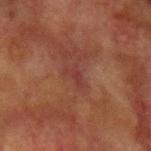Notes:
– notes: no biopsy performed (imaged during a skin exam)
– body site: the left upper arm
– imaging modality: total-body-photography crop, ~15 mm field of view
– subject: male, about 75 years old
– size: about 2.5 mm
– image-analysis metrics: a lesion color around L≈31 a*≈23 b*≈22 in CIELAB and about 5 CIELAB-L* units darker than the surrounding skin; a border-irregularity index near 4.5/10, a within-lesion color-variation index near 1/10, and peripheral color asymmetry of about 0